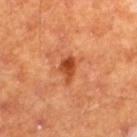workup: catalogued during a skin exam; not biopsied | lighting: cross-polarized | patient: male, about 60 years old | location: the left thigh | image: total-body-photography crop, ~15 mm field of view | lesion diameter: ~3 mm (longest diameter).